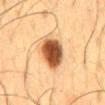Impression: The lesion was tiled from a total-body skin photograph and was not biopsied. Context: Cropped from a whole-body photographic skin survey; the tile spans about 15 mm. An algorithmic analysis of the crop reported border irregularity of about 1.5 on a 0–10 scale and a peripheral color-asymmetry measure near 2. The analysis additionally found a nevus-likeness score of about 100/100 and lesion-presence confidence of about 100/100. From the mid back. A male patient approximately 60 years of age. Measured at roughly 5 mm in maximum diameter. The tile uses cross-polarized illumination.